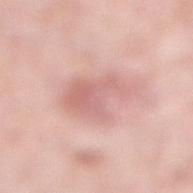Acquisition and patient details: Measured at roughly 5 mm in maximum diameter. The tile uses white-light illumination. Automated image analysis of the tile measured a lesion color around L≈66 a*≈23 b*≈24 in CIELAB, a lesion–skin lightness drop of about 9, and a normalized lesion–skin contrast near 5.5. From the leg. A 15 mm close-up tile from a total-body photography series done for melanoma screening. The subject is a female in their mid- to late 50s.